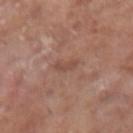workup = total-body-photography surveillance lesion; no biopsy | acquisition = ~15 mm tile from a whole-body skin photo | subject = male, aged approximately 75 | site = the right upper arm.